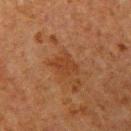Captured during whole-body skin photography for melanoma surveillance; the lesion was not biopsied.
A male subject, aged 58 to 62.
The lesion is on the left upper arm.
Cropped from a total-body skin-imaging series; the visible field is about 15 mm.